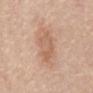Part of a total-body skin-imaging series; this lesion was reviewed on a skin check and was not flagged for biopsy. This image is a 15 mm lesion crop taken from a total-body photograph. A male subject, in their 80s. Longest diameter approximately 5 mm. The lesion is on the back.